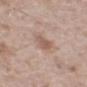| field | value |
|---|---|
| workup | imaged on a skin check; not biopsied |
| patient | male, aged 63–67 |
| body site | the chest |
| image source | ~15 mm tile from a whole-body skin photo |
| size | ≈3 mm |
| lighting | white-light illumination |